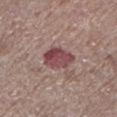Q: Is there a histopathology result?
A: imaged on a skin check; not biopsied
Q: What lighting was used for the tile?
A: white-light
Q: What kind of image is this?
A: ~15 mm crop, total-body skin-cancer survey
Q: Patient demographics?
A: male, aged 63 to 67
Q: Lesion size?
A: ~3.5 mm (longest diameter)
Q: What is the anatomic site?
A: the left lower leg